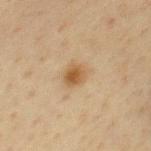Assessment:
Recorded during total-body skin imaging; not selected for excision or biopsy.
Image and clinical context:
Imaged with cross-polarized lighting. A close-up tile cropped from a whole-body skin photograph, about 15 mm across. A male patient, aged 58 to 62. The lesion is located on the chest. Automated tile analysis of the lesion measured a border-irregularity rating of about 2/10, internal color variation of about 4.5 on a 0–10 scale, and a peripheral color-asymmetry measure near 1.5.